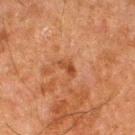A male subject, aged 78–82. A 15 mm close-up tile from a total-body photography series done for melanoma screening. Located on the right thigh. Approximately 2.5 mm at its widest.